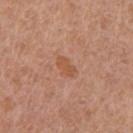Q: How was the tile lit?
A: white-light
Q: Patient demographics?
A: female, aged around 55
Q: What kind of image is this?
A: ~15 mm tile from a whole-body skin photo
Q: Lesion size?
A: ≈3 mm
Q: Where on the body is the lesion?
A: the left thigh